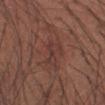acquisition — ~15 mm tile from a whole-body skin photo | subject — male, approximately 35 years of age | image-analysis metrics — a footprint of about 5 mm², an outline eccentricity of about 0.9 (0 = round, 1 = elongated), and a shape-asymmetry score of about 0.4 (0 = symmetric); border irregularity of about 5 on a 0–10 scale, a within-lesion color-variation index near 1.5/10, and a peripheral color-asymmetry measure near 0.5; a nevus-likeness score of about 0/100 and a detector confidence of about 85 out of 100 that the crop contains a lesion | illumination — white-light illumination | lesion size — ~3.5 mm (longest diameter) | body site — the right forearm.